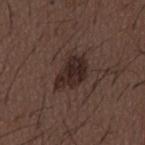biopsy_status: not biopsied; imaged during a skin examination
site: back
image:
  source: total-body photography crop
  field_of_view_mm: 15
patient:
  sex: male
  age_approx: 50